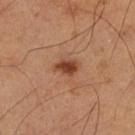Clinical impression: Part of a total-body skin-imaging series; this lesion was reviewed on a skin check and was not flagged for biopsy. Image and clinical context: On the leg. Longest diameter approximately 3 mm. A 15 mm close-up tile from a total-body photography series done for melanoma screening. A male patient about 60 years old. Automated image analysis of the tile measured an area of roughly 4 mm², an eccentricity of roughly 0.75, and a symmetry-axis asymmetry near 0.25. And it measured an average lesion color of about L≈42 a*≈25 b*≈32 (CIELAB), a lesion–skin lightness drop of about 13, and a lesion-to-skin contrast of about 9.5 (normalized; higher = more distinct). It also reported a border-irregularity rating of about 2.5/10 and a color-variation rating of about 2/10. Captured under cross-polarized illumination.